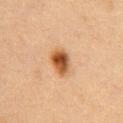Notes:
* biopsy status — imaged on a skin check; not biopsied
* tile lighting — cross-polarized
* site — the chest
* patient — female, aged around 40
* size — about 3.5 mm
* acquisition — 15 mm crop, total-body photography